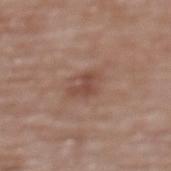– acquisition: ~15 mm crop, total-body skin-cancer survey
– location: the upper back
– tile lighting: white-light
– patient: female, in their mid- to late 60s
– size: ~3.5 mm (longest diameter)